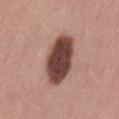Context: The patient is a female in their mid- to late 50s. A roughly 15 mm field-of-view crop from a total-body skin photograph. The lesion is located on the back. The lesion's longest dimension is about 6.5 mm.